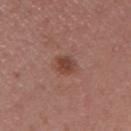* biopsy status — total-body-photography surveillance lesion; no biopsy
* lighting — white-light illumination
* lesion diameter — ≈3 mm
* imaging modality — ~15 mm crop, total-body skin-cancer survey
* anatomic site — the right upper arm
* subject — male, approximately 40 years of age